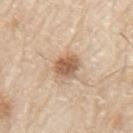Case summary:
* image source · total-body-photography crop, ~15 mm field of view
* illumination · white-light illumination
* image-analysis metrics · a footprint of about 7 mm² and a shape eccentricity near 0.6; border irregularity of about 1.5 on a 0–10 scale and a within-lesion color-variation index near 3.5/10; a classifier nevus-likeness of about 85/100 and a lesion-detection confidence of about 100/100
* lesion diameter · ~3.5 mm (longest diameter)
* patient · male, about 80 years old
* location · the left upper arm